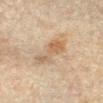Findings:
– workup: no biopsy performed (imaged during a skin exam)
– patient: female, approximately 40 years of age
– lighting: cross-polarized illumination
– diameter: ~4.5 mm (longest diameter)
– image-analysis metrics: a mean CIELAB color near L≈52 a*≈14 b*≈29, a lesion–skin lightness drop of about 7, and a normalized border contrast of about 6; border irregularity of about 6 on a 0–10 scale, a color-variation rating of about 4/10, and radial color variation of about 1; an automated nevus-likeness rating near 10 out of 100 and a detector confidence of about 100 out of 100 that the crop contains a lesion
– site: the arm
– image: ~15 mm tile from a whole-body skin photo